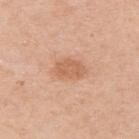Impression:
Part of a total-body skin-imaging series; this lesion was reviewed on a skin check and was not flagged for biopsy.
Image and clinical context:
The subject is a female aged 38 to 42. A 15 mm crop from a total-body photograph taken for skin-cancer surveillance. The lesion is on the right upper arm. The tile uses white-light illumination. Approximately 3.5 mm at its widest. The total-body-photography lesion software estimated an average lesion color of about L≈61 a*≈23 b*≈34 (CIELAB), roughly 9 lightness units darker than nearby skin, and a lesion-to-skin contrast of about 6 (normalized; higher = more distinct). And it measured a border-irregularity rating of about 3/10, a color-variation rating of about 2/10, and a peripheral color-asymmetry measure near 0.5. And it measured a classifier nevus-likeness of about 30/100 and a detector confidence of about 100 out of 100 that the crop contains a lesion.